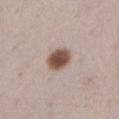| key | value |
|---|---|
| biopsy status | catalogued during a skin exam; not biopsied |
| anatomic site | the left lower leg |
| image source | ~15 mm crop, total-body skin-cancer survey |
| subject | female, aged around 40 |